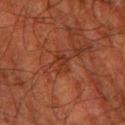Part of a total-body skin-imaging series; this lesion was reviewed on a skin check and was not flagged for biopsy.
About 2.5 mm across.
The lesion-visualizer software estimated a footprint of about 4 mm² and a symmetry-axis asymmetry near 0.25. It also reported a border-irregularity index near 3/10, internal color variation of about 2.5 on a 0–10 scale, and a peripheral color-asymmetry measure near 1.
On the left lower leg.
A 15 mm close-up tile from a total-body photography series done for melanoma screening.
The patient is a male aged 78–82.
Captured under cross-polarized illumination.